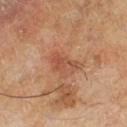notes: imaged on a skin check; not biopsied
patient: male, in their mid-60s
lighting: cross-polarized illumination
automated metrics: an eccentricity of roughly 0.8 and two-axis asymmetry of about 0.5; an average lesion color of about L≈51 a*≈26 b*≈33 (CIELAB), about 8 CIELAB-L* units darker than the surrounding skin, and a lesion-to-skin contrast of about 5.5 (normalized; higher = more distinct); border irregularity of about 6 on a 0–10 scale and radial color variation of about 1
lesion size: ~3.5 mm (longest diameter)
body site: the left lower leg
image: ~15 mm tile from a whole-body skin photo